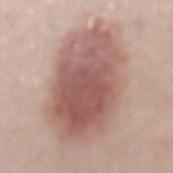Assessment: Captured during whole-body skin photography for melanoma surveillance; the lesion was not biopsied. Clinical summary: The lesion's longest dimension is about 11.5 mm. The tile uses white-light illumination. A female subject roughly 50 years of age. On the mid back. A close-up tile cropped from a whole-body skin photograph, about 15 mm across.